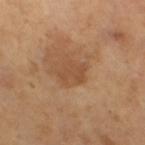The lesion is located on the right thigh.
The tile uses cross-polarized illumination.
The recorded lesion diameter is about 3 mm.
A female patient, aged 53 to 57.
Cropped from a total-body skin-imaging series; the visible field is about 15 mm.
The lesion-visualizer software estimated an area of roughly 5.5 mm², an eccentricity of roughly 0.65, and a shape-asymmetry score of about 0.25 (0 = symmetric). The software also gave a mean CIELAB color near L≈50 a*≈21 b*≈34, about 7 CIELAB-L* units darker than the surrounding skin, and a normalized border contrast of about 5.